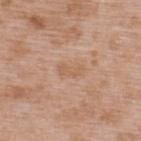Findings:
- notes: catalogued during a skin exam; not biopsied
- site: the upper back
- subject: male, in their 50s
- acquisition: ~15 mm tile from a whole-body skin photo
- tile lighting: white-light illumination